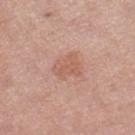Part of a total-body skin-imaging series; this lesion was reviewed on a skin check and was not flagged for biopsy.
The lesion is located on the right lower leg.
Longest diameter approximately 3 mm.
Captured under white-light illumination.
An algorithmic analysis of the crop reported an average lesion color of about L≈59 a*≈23 b*≈29 (CIELAB), about 7 CIELAB-L* units darker than the surrounding skin, and a lesion-to-skin contrast of about 5 (normalized; higher = more distinct).
A female subject roughly 70 years of age.
A region of skin cropped from a whole-body photographic capture, roughly 15 mm wide.